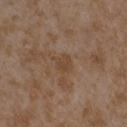Clinical impression: Part of a total-body skin-imaging series; this lesion was reviewed on a skin check and was not flagged for biopsy. Background: The lesion is located on the right forearm. The lesion's longest dimension is about 2.5 mm. A female patient, aged approximately 35. Automated image analysis of the tile measured a lesion area of about 4.5 mm², an outline eccentricity of about 0.45 (0 = round, 1 = elongated), and a symmetry-axis asymmetry near 0.25. The analysis additionally found an average lesion color of about L≈44 a*≈17 b*≈30 (CIELAB), a lesion–skin lightness drop of about 6, and a normalized lesion–skin contrast near 5.5. It also reported a classifier nevus-likeness of about 0/100. Cropped from a whole-body photographic skin survey; the tile spans about 15 mm.